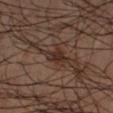Imaged during a routine full-body skin examination; the lesion was not biopsied and no histopathology is available. A male subject, aged 48–52. The lesion is located on the left arm. The recorded lesion diameter is about 4.5 mm. A roughly 15 mm field-of-view crop from a total-body skin photograph. This is a cross-polarized tile. Automated image analysis of the tile measured a lesion area of about 5 mm², an outline eccentricity of about 0.95 (0 = round, 1 = elongated), and a symmetry-axis asymmetry near 0.25. And it measured a normalized lesion–skin contrast near 8.5. The software also gave a border-irregularity index near 3.5/10 and a within-lesion color-variation index near 1/10.